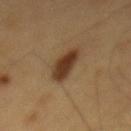Part of a total-body skin-imaging series; this lesion was reviewed on a skin check and was not flagged for biopsy.
From the mid back.
Measured at roughly 5.5 mm in maximum diameter.
The subject is a male in their 60s.
This image is a 15 mm lesion crop taken from a total-body photograph.